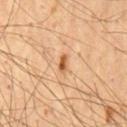Recorded during total-body skin imaging; not selected for excision or biopsy. This image is a 15 mm lesion crop taken from a total-body photograph. The patient is a male aged 53–57. On the chest.